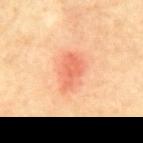The lesion was tiled from a total-body skin photograph and was not biopsied.
On the back.
About 4.5 mm across.
A male subject, aged around 60.
This image is a 15 mm lesion crop taken from a total-body photograph.
Imaged with cross-polarized lighting.